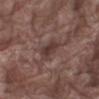Q: What are the patient's age and sex?
A: male, aged 78 to 82
Q: Where on the body is the lesion?
A: the leg
Q: How large is the lesion?
A: about 4 mm
Q: Automated lesion metrics?
A: a footprint of about 5.5 mm² and an eccentricity of roughly 0.9; an automated nevus-likeness rating near 5 out of 100 and a detector confidence of about 75 out of 100 that the crop contains a lesion
Q: What kind of image is this?
A: 15 mm crop, total-body photography